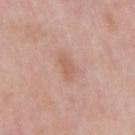| key | value |
|---|---|
| site | the arm |
| illumination | white-light illumination |
| subject | male, roughly 55 years of age |
| size | ~2.5 mm (longest diameter) |
| image | 15 mm crop, total-body photography |